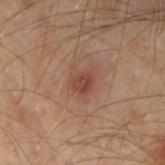Assessment: The lesion was photographed on a routine skin check and not biopsied; there is no pathology result. Image and clinical context: A lesion tile, about 15 mm wide, cut from a 3D total-body photograph. The lesion is located on the left forearm. A male subject, aged 48–52.